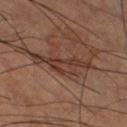Q: Was a biopsy performed?
A: total-body-photography surveillance lesion; no biopsy
Q: What are the patient's age and sex?
A: male, aged 58–62
Q: What is the anatomic site?
A: the leg
Q: What kind of image is this?
A: 15 mm crop, total-body photography
Q: What did automated image analysis measure?
A: a lesion color around L≈36 a*≈18 b*≈24 in CIELAB, roughly 8 lightness units darker than nearby skin, and a normalized lesion–skin contrast near 7; an automated nevus-likeness rating near 0 out of 100 and a detector confidence of about 55 out of 100 that the crop contains a lesion